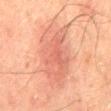Part of a total-body skin-imaging series; this lesion was reviewed on a skin check and was not flagged for biopsy. A male subject aged around 65. From the back. Imaged with cross-polarized lighting. Automated tile analysis of the lesion measured a lesion area of about 29 mm², an outline eccentricity of about 0.75 (0 = round, 1 = elongated), and a symmetry-axis asymmetry near 0.2. The software also gave a mean CIELAB color near L≈52 a*≈23 b*≈28, a lesion–skin lightness drop of about 7, and a normalized border contrast of about 5.5. The software also gave a classifier nevus-likeness of about 15/100 and a detector confidence of about 100 out of 100 that the crop contains a lesion. The lesion's longest dimension is about 7.5 mm. Cropped from a whole-body photographic skin survey; the tile spans about 15 mm.